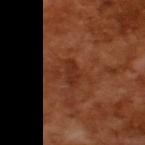The lesion was photographed on a routine skin check and not biopsied; there is no pathology result.
A 15 mm close-up tile from a total-body photography series done for melanoma screening.
A male patient aged approximately 65.